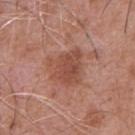This lesion was catalogued during total-body skin photography and was not selected for biopsy.
A male patient, in their mid-70s.
Located on the chest.
A close-up tile cropped from a whole-body skin photograph, about 15 mm across.
An algorithmic analysis of the crop reported lesion-presence confidence of about 100/100.